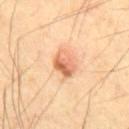  site: chest
  image:
    source: total-body photography crop
    field_of_view_mm: 15
  patient:
    sex: male
    age_approx: 60
  lighting: cross-polarized